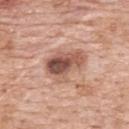No biopsy was performed on this lesion — it was imaged during a full skin examination and was not determined to be concerning. The lesion is on the back. The subject is a female roughly 60 years of age. Cropped from a whole-body photographic skin survey; the tile spans about 15 mm. This is a white-light tile.